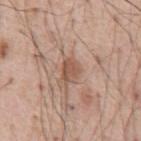  biopsy_status: not biopsied; imaged during a skin examination
  image:
    source: total-body photography crop
    field_of_view_mm: 15
  site: abdomen
  patient:
    sex: male
    age_approx: 55
  lesion_size:
    long_diameter_mm_approx: 3.0